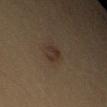This lesion was catalogued during total-body skin photography and was not selected for biopsy. The total-body-photography lesion software estimated a border-irregularity rating of about 3.5/10, internal color variation of about 1.5 on a 0–10 scale, and radial color variation of about 0.5. The tile uses cross-polarized illumination. The subject is a male roughly 35 years of age. The lesion is on the arm. This image is a 15 mm lesion crop taken from a total-body photograph. The lesion's longest dimension is about 2.5 mm.